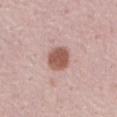Part of a total-body skin-imaging series; this lesion was reviewed on a skin check and was not flagged for biopsy.
A male subject in their 60s.
This image is a 15 mm lesion crop taken from a total-body photograph.
Imaged with white-light lighting.
Automated tile analysis of the lesion measured a lesion color around L≈55 a*≈23 b*≈26 in CIELAB and roughly 14 lightness units darker than nearby skin. The analysis additionally found a border-irregularity index near 1.5/10, internal color variation of about 2.5 on a 0–10 scale, and radial color variation of about 0.5. It also reported a nevus-likeness score of about 100/100 and lesion-presence confidence of about 100/100.
On the abdomen.
Measured at roughly 3 mm in maximum diameter.